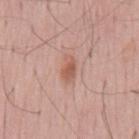| field | value |
|---|---|
| patient | male, in their mid-50s |
| diameter | ~3 mm (longest diameter) |
| image-analysis metrics | a lesion area of about 4.5 mm², a shape eccentricity near 0.85, and two-axis asymmetry of about 0.25; a mean CIELAB color near L≈57 a*≈23 b*≈29, about 10 CIELAB-L* units darker than the surrounding skin, and a normalized lesion–skin contrast near 7; a border-irregularity rating of about 2.5/10, a color-variation rating of about 2/10, and radial color variation of about 0.5; a classifier nevus-likeness of about 70/100 |
| illumination | white-light |
| acquisition | 15 mm crop, total-body photography |